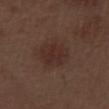notes: total-body-photography surveillance lesion; no biopsy
image: ~15 mm crop, total-body skin-cancer survey
automated metrics: a nevus-likeness score of about 30/100 and a lesion-detection confidence of about 100/100
lesion diameter: ≈4 mm
subject: male, aged 68 to 72
illumination: white-light
anatomic site: the left thigh